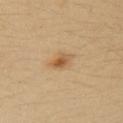Recorded during total-body skin imaging; not selected for excision or biopsy.
The lesion's longest dimension is about 3 mm.
The lesion is on the right upper arm.
Cropped from a total-body skin-imaging series; the visible field is about 15 mm.
The tile uses cross-polarized illumination.
A female patient, approximately 35 years of age.